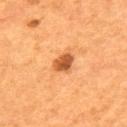Notes:
* notes — imaged on a skin check; not biopsied
* automated lesion analysis — a footprint of about 4.5 mm², a shape eccentricity near 0.6, and a shape-asymmetry score of about 0.25 (0 = symmetric); about 15 CIELAB-L* units darker than the surrounding skin and a normalized border contrast of about 9.5; radial color variation of about 1; a nevus-likeness score of about 90/100 and lesion-presence confidence of about 100/100
* patient — male, in their mid- to late 50s
* body site — the upper back
* lighting — cross-polarized
* size — ~2.5 mm (longest diameter)
* image — ~15 mm crop, total-body skin-cancer survey